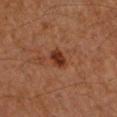Q: Lesion location?
A: the left forearm
Q: How large is the lesion?
A: about 2.5 mm
Q: How was this image acquired?
A: total-body-photography crop, ~15 mm field of view
Q: What are the patient's age and sex?
A: male, in their mid- to late 80s or older